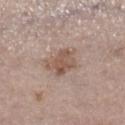Findings:
• biopsy status: no biopsy performed (imaged during a skin exam)
• site: the right lower leg
• subject: female, aged approximately 65
• image source: total-body-photography crop, ~15 mm field of view
• TBP lesion metrics: an area of roughly 8.5 mm², an eccentricity of roughly 0.75, and two-axis asymmetry of about 0.3; a nevus-likeness score of about 25/100 and lesion-presence confidence of about 100/100